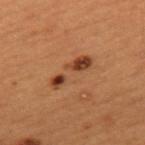biopsy status = catalogued during a skin exam; not biopsied
location = the mid back
image = ~15 mm tile from a whole-body skin photo
patient = male, aged 48 to 52
illumination = cross-polarized
automated lesion analysis = an eccentricity of roughly 0.85 and a symmetry-axis asymmetry near 0.2; a mean CIELAB color near L≈35 a*≈19 b*≈28, a lesion–skin lightness drop of about 8, and a normalized lesion–skin contrast near 7; a detector confidence of about 100 out of 100 that the crop contains a lesion